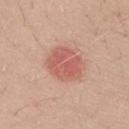{"biopsy_status": "not biopsied; imaged during a skin examination", "image": {"source": "total-body photography crop", "field_of_view_mm": 15}, "lesion_size": {"long_diameter_mm_approx": 4.0}, "site": "arm", "automated_metrics": {"eccentricity": 0.5, "shape_asymmetry": 0.25, "nevus_likeness_0_100": 35, "lesion_detection_confidence_0_100": 100}, "patient": {"sex": "male", "age_approx": 30}}